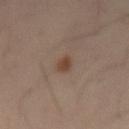| key | value |
|---|---|
| workup | catalogued during a skin exam; not biopsied |
| image source | total-body-photography crop, ~15 mm field of view |
| lighting | cross-polarized illumination |
| patient | male, aged 38–42 |
| automated metrics | an eccentricity of roughly 0.85 and a symmetry-axis asymmetry near 0.2; a border-irregularity rating of about 2/10 and peripheral color asymmetry of about 0.5 |
| size | ~3 mm (longest diameter) |
| body site | the mid back |